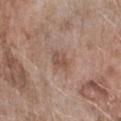notes: no biopsy performed (imaged during a skin exam) | tile lighting: white-light | image-analysis metrics: a footprint of about 4 mm², an eccentricity of roughly 0.75, and a shape-asymmetry score of about 0.25 (0 = symmetric); border irregularity of about 2.5 on a 0–10 scale, internal color variation of about 2.5 on a 0–10 scale, and a peripheral color-asymmetry measure near 1; a nevus-likeness score of about 0/100 and lesion-presence confidence of about 100/100 | anatomic site: the right forearm | diameter: ≈2.5 mm | image source: ~15 mm tile from a whole-body skin photo | patient: female, in their mid- to late 70s.